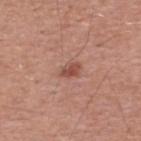Imaged during a routine full-body skin examination; the lesion was not biopsied and no histopathology is available. The tile uses white-light illumination. Longest diameter approximately 2.5 mm. The subject is a male aged around 55. From the upper back. This image is a 15 mm lesion crop taken from a total-body photograph.